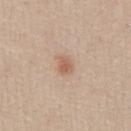The lesion was tiled from a total-body skin photograph and was not biopsied.
Imaged with white-light lighting.
A 15 mm crop from a total-body photograph taken for skin-cancer surveillance.
Automated image analysis of the tile measured a lesion area of about 4 mm². And it measured an automated nevus-likeness rating near 80 out of 100 and a detector confidence of about 100 out of 100 that the crop contains a lesion.
On the front of the torso.
The patient is a male aged 33 to 37.